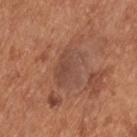workup: catalogued during a skin exam; not biopsied
body site: the upper back
lesion diameter: ~4.5 mm (longest diameter)
lighting: white-light
acquisition: 15 mm crop, total-body photography
image-analysis metrics: a border-irregularity rating of about 3/10, a within-lesion color-variation index near 3.5/10, and peripheral color asymmetry of about 1.5
subject: male, aged approximately 55